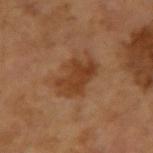Imaged during a routine full-body skin examination; the lesion was not biopsied and no histopathology is available. Longest diameter approximately 5 mm. A close-up tile cropped from a whole-body skin photograph, about 15 mm across. Captured under cross-polarized illumination. On the arm. The lesion-visualizer software estimated a footprint of about 13 mm², a shape eccentricity near 0.75, and a shape-asymmetry score of about 0.3 (0 = symmetric). The analysis additionally found an average lesion color of about L≈39 a*≈21 b*≈33 (CIELAB), roughly 8 lightness units darker than nearby skin, and a normalized border contrast of about 7.5. The software also gave a border-irregularity rating of about 3.5/10, internal color variation of about 3.5 on a 0–10 scale, and radial color variation of about 1.5. A male patient roughly 65 years of age.